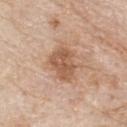• workup — no biopsy performed (imaged during a skin exam)
• subject — male, aged approximately 85 or older
• lesion diameter — ~4.5 mm (longest diameter)
• automated lesion analysis — a lesion area of about 10 mm², a shape eccentricity near 0.7, and a shape-asymmetry score of about 0.25 (0 = symmetric); lesion-presence confidence of about 100/100
• image source — total-body-photography crop, ~15 mm field of view
• tile lighting — white-light
• location — the upper back